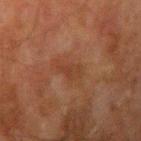Findings:
• notes: imaged on a skin check; not biopsied
• site: the left arm
• lighting: cross-polarized illumination
• patient: male, about 60 years old
• image: 15 mm crop, total-body photography